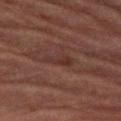The lesion was tiled from a total-body skin photograph and was not biopsied.